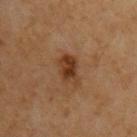The lesion was photographed on a routine skin check and not biopsied; there is no pathology result. Captured under cross-polarized illumination. A male subject about 60 years old. A roughly 15 mm field-of-view crop from a total-body skin photograph. The lesion is located on the right upper arm.